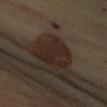Clinical impression:
Part of a total-body skin-imaging series; this lesion was reviewed on a skin check and was not flagged for biopsy.
Acquisition and patient details:
The lesion is located on the right upper arm. Measured at roughly 5.5 mm in maximum diameter. Captured under cross-polarized illumination. This image is a 15 mm lesion crop taken from a total-body photograph. The patient is a female in their 60s.